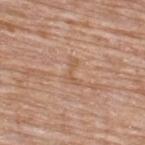biopsy_status: not biopsied; imaged during a skin examination
image:
  source: total-body photography crop
  field_of_view_mm: 15
site: upper back
patient:
  sex: male
  age_approx: 65
lighting: white-light